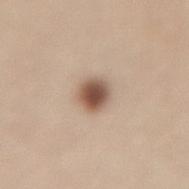Q: Was this lesion biopsied?
A: catalogued during a skin exam; not biopsied
Q: What are the patient's age and sex?
A: female, aged approximately 40
Q: How was this image acquired?
A: 15 mm crop, total-body photography
Q: Lesion location?
A: the lower back